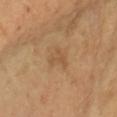Captured during whole-body skin photography for melanoma surveillance; the lesion was not biopsied.
The subject is a female in their mid-50s.
The lesion is on the left forearm.
A 15 mm close-up extracted from a 3D total-body photography capture.
The total-body-photography lesion software estimated a footprint of about 3 mm² and a shape-asymmetry score of about 0.6 (0 = symmetric). The software also gave an average lesion color of about L≈53 a*≈19 b*≈37 (CIELAB) and a lesion-to-skin contrast of about 5 (normalized; higher = more distinct). It also reported a border-irregularity rating of about 8/10, internal color variation of about 0 on a 0–10 scale, and a peripheral color-asymmetry measure near 0. And it measured a classifier nevus-likeness of about 0/100.
Captured under cross-polarized illumination.